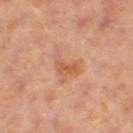| feature | finding |
|---|---|
| biopsy status | no biopsy performed (imaged during a skin exam) |
| image-analysis metrics | a lesion-to-skin contrast of about 5 (normalized; higher = more distinct); a within-lesion color-variation index near 3.5/10 and radial color variation of about 1 |
| lighting | cross-polarized |
| size | ≈5 mm |
| image source | ~15 mm tile from a whole-body skin photo |
| site | the right thigh |
| patient | female, about 40 years old |